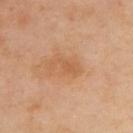No biopsy was performed on this lesion — it was imaged during a full skin examination and was not determined to be concerning. The lesion is located on the back. The recorded lesion diameter is about 3.5 mm. The subject is a female aged 38–42. The lesion-visualizer software estimated an average lesion color of about L≈59 a*≈23 b*≈38 (CIELAB), about 7 CIELAB-L* units darker than the surrounding skin, and a normalized border contrast of about 5.5. A roughly 15 mm field-of-view crop from a total-body skin photograph. The tile uses cross-polarized illumination.